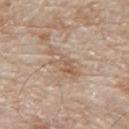{
  "biopsy_status": "not biopsied; imaged during a skin examination",
  "patient": {
    "sex": "male",
    "age_approx": 80
  },
  "lighting": "white-light",
  "automated_metrics": {
    "cielab_L": 59,
    "cielab_a": 15,
    "cielab_b": 29,
    "vs_skin_darker_L": 7.0,
    "vs_skin_contrast_norm": 5.5
  },
  "image": {
    "source": "total-body photography crop",
    "field_of_view_mm": 15
  },
  "site": "back"
}